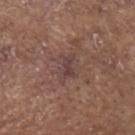Assessment:
Part of a total-body skin-imaging series; this lesion was reviewed on a skin check and was not flagged for biopsy.
Context:
The lesion is on the head or neck. This image is a 15 mm lesion crop taken from a total-body photograph. This is a white-light tile. A male subject aged around 80. The lesion-visualizer software estimated a shape eccentricity near 0.65 and two-axis asymmetry of about 0.35. And it measured an automated nevus-likeness rating near 0 out of 100 and a detector confidence of about 70 out of 100 that the crop contains a lesion.